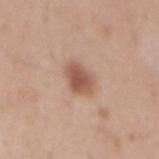{
  "biopsy_status": "not biopsied; imaged during a skin examination",
  "image": {
    "source": "total-body photography crop",
    "field_of_view_mm": 15
  },
  "lesion_size": {
    "long_diameter_mm_approx": 3.5
  },
  "site": "mid back",
  "lighting": "white-light",
  "automated_metrics": {
    "cielab_L": 54,
    "cielab_a": 21,
    "cielab_b": 29,
    "vs_skin_darker_L": 12.0,
    "vs_skin_contrast_norm": 8.5,
    "nevus_likeness_0_100": 95,
    "lesion_detection_confidence_0_100": 100
  },
  "patient": {
    "sex": "male",
    "age_approx": 55
  }
}